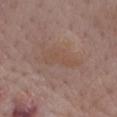Clinical summary:
Captured under white-light illumination. The patient is a female aged approximately 70. Cropped from a whole-body photographic skin survey; the tile spans about 15 mm. On the back.